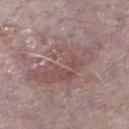Clinical summary: A lesion tile, about 15 mm wide, cut from a 3D total-body photograph. The tile uses white-light illumination. The lesion is on the right lower leg. A male patient aged approximately 75.